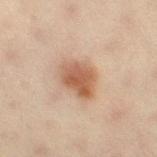About 4.5 mm across. A female patient aged around 45. Automated image analysis of the tile measured border irregularity of about 2.5 on a 0–10 scale, a within-lesion color-variation index near 4/10, and peripheral color asymmetry of about 1.5. It also reported a classifier nevus-likeness of about 95/100. From the right thigh. A close-up tile cropped from a whole-body skin photograph, about 15 mm across.